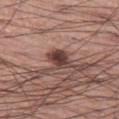notes: catalogued during a skin exam; not biopsied | site: the left thigh | subject: male, aged 58 to 62 | acquisition: 15 mm crop, total-body photography | automated metrics: an area of roughly 5.5 mm² and an eccentricity of roughly 0.65; a lesion color around L≈40 a*≈20 b*≈21 in CIELAB, about 15 CIELAB-L* units darker than the surrounding skin, and a lesion-to-skin contrast of about 11.5 (normalized; higher = more distinct) | illumination: white-light.